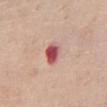Q: Was a biopsy performed?
A: no biopsy performed (imaged during a skin exam)
Q: What is the anatomic site?
A: the chest
Q: Patient demographics?
A: female, about 50 years old
Q: How large is the lesion?
A: about 2.5 mm
Q: How was this image acquired?
A: total-body-photography crop, ~15 mm field of view
Q: How was the tile lit?
A: white-light illumination